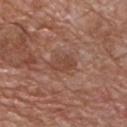{"biopsy_status": "not biopsied; imaged during a skin examination", "image": {"source": "total-body photography crop", "field_of_view_mm": 15}, "site": "chest", "patient": {"sex": "male", "age_approx": 70}}